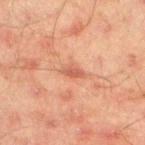Case summary:
- notes: catalogued during a skin exam; not biopsied
- anatomic site: the left lower leg
- patient: male, aged approximately 45
- acquisition: 15 mm crop, total-body photography